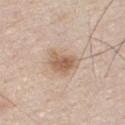notes: imaged on a skin check; not biopsied
image: total-body-photography crop, ~15 mm field of view
illumination: white-light
image-analysis metrics: internal color variation of about 3.5 on a 0–10 scale and peripheral color asymmetry of about 1; a classifier nevus-likeness of about 80/100 and a detector confidence of about 100 out of 100 that the crop contains a lesion
body site: the chest
subject: male, aged around 45
diameter: about 3.5 mm